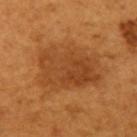Assessment:
This lesion was catalogued during total-body skin photography and was not selected for biopsy.
Background:
From the arm. A roughly 15 mm field-of-view crop from a total-body skin photograph. A male subject, roughly 65 years of age.